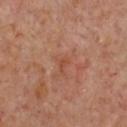Part of a total-body skin-imaging series; this lesion was reviewed on a skin check and was not flagged for biopsy. Automated tile analysis of the lesion measured roughly 6 lightness units darker than nearby skin. It also reported a border-irregularity rating of about 3.5/10, a within-lesion color-variation index near 0/10, and peripheral color asymmetry of about 0. On the front of the torso. A roughly 15 mm field-of-view crop from a total-body skin photograph. Approximately 1 mm at its widest. A female patient aged 58 to 62.